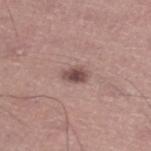biopsy_status: not biopsied; imaged during a skin examination
automated_metrics:
  area_mm2_approx: 4.0
  eccentricity: 0.8
  shape_asymmetry: 0.2
lighting: white-light
site: left lower leg
image:
  source: total-body photography crop
  field_of_view_mm: 15
patient:
  sex: male
  age_approx: 45
lesion_size:
  long_diameter_mm_approx: 2.5